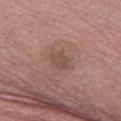Q: Was a biopsy performed?
A: catalogued during a skin exam; not biopsied
Q: What are the patient's age and sex?
A: male, roughly 75 years of age
Q: What is the imaging modality?
A: ~15 mm tile from a whole-body skin photo
Q: What lighting was used for the tile?
A: white-light illumination
Q: How large is the lesion?
A: about 2.5 mm
Q: What did automated image analysis measure?
A: an area of roughly 4.5 mm² and a shape eccentricity near 0.6; a classifier nevus-likeness of about 0/100 and lesion-presence confidence of about 100/100
Q: Where on the body is the lesion?
A: the left lower leg